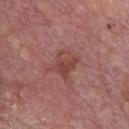| field | value |
|---|---|
| follow-up | no biopsy performed (imaged during a skin exam) |
| image | ~15 mm crop, total-body skin-cancer survey |
| size | ~3 mm (longest diameter) |
| illumination | white-light |
| patient | male, aged 73 to 77 |
| location | the chest |